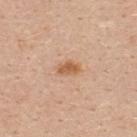Recorded during total-body skin imaging; not selected for excision or biopsy. Located on the upper back. The lesion's longest dimension is about 2.5 mm. The patient is a female approximately 40 years of age. A 15 mm crop from a total-body photograph taken for skin-cancer surveillance. Captured under white-light illumination. Automated tile analysis of the lesion measured a border-irregularity rating of about 2/10 and a peripheral color-asymmetry measure near 1.